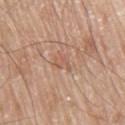Captured during whole-body skin photography for melanoma surveillance; the lesion was not biopsied.
A male subject, approximately 80 years of age.
Located on the chest.
Captured under white-light illumination.
Cropped from a total-body skin-imaging series; the visible field is about 15 mm.